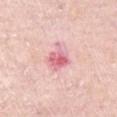Clinical impression:
Imaged during a routine full-body skin examination; the lesion was not biopsied and no histopathology is available.
Acquisition and patient details:
The lesion is located on the chest. A 15 mm crop from a total-body photograph taken for skin-cancer surveillance. A male patient, in their mid-70s.